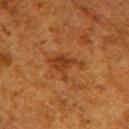  biopsy_status: not biopsied; imaged during a skin examination
  patient:
    sex: female
    age_approx: 50
  lesion_size:
    long_diameter_mm_approx: 4.0
  image:
    source: total-body photography crop
    field_of_view_mm: 15
  lighting: cross-polarized
  site: upper back
  automated_metrics:
    area_mm2_approx: 6.5
    eccentricity: 0.7
    cielab_L: 32
    cielab_a: 22
    cielab_b: 33
    vs_skin_darker_L: 7.0
    vs_skin_contrast_norm: 7.0
    border_irregularity_0_10: 5.0
    color_variation_0_10: 2.0
    peripheral_color_asymmetry: 1.0